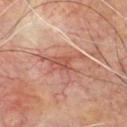| field | value |
|---|---|
| notes | total-body-photography surveillance lesion; no biopsy |
| anatomic site | the chest |
| subject | male, aged 58–62 |
| illumination | cross-polarized |
| image | total-body-photography crop, ~15 mm field of view |